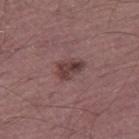{
  "biopsy_status": "not biopsied; imaged during a skin examination",
  "image": {
    "source": "total-body photography crop",
    "field_of_view_mm": 15
  },
  "lighting": "white-light",
  "patient": {
    "sex": "male",
    "age_approx": 60
  },
  "site": "leg",
  "lesion_size": {
    "long_diameter_mm_approx": 3.5
  }
}